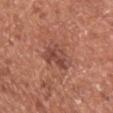Notes:
- follow-up — catalogued during a skin exam; not biopsied
- tile lighting — white-light illumination
- patient — male, about 75 years old
- location — the chest
- lesion size — ≈4 mm
- image — ~15 mm tile from a whole-body skin photo
- automated metrics — a lesion area of about 8 mm², a shape eccentricity near 0.65, and a symmetry-axis asymmetry near 0.4; a mean CIELAB color near L≈47 a*≈25 b*≈27, about 9 CIELAB-L* units darker than the surrounding skin, and a normalized border contrast of about 7; an automated nevus-likeness rating near 0 out of 100 and a detector confidence of about 100 out of 100 that the crop contains a lesion